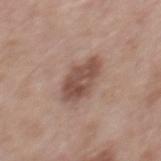<lesion>
<lighting>white-light</lighting>
<lesion_size>
  <long_diameter_mm_approx>5.0</long_diameter_mm_approx>
</lesion_size>
<automated_metrics>
  <cielab_L>50</cielab_L>
  <cielab_a>19</cielab_a>
  <cielab_b>24</cielab_b>
  <vs_skin_contrast_norm>8.5</vs_skin_contrast_norm>
  <border_irregularity_0_10>2.5</border_irregularity_0_10>
  <color_variation_0_10>3.5</color_variation_0_10>
  <peripheral_color_asymmetry>1.5</peripheral_color_asymmetry>
</automated_metrics>
<site>mid back</site>
<patient>
  <sex>male</sex>
  <age_approx>55</age_approx>
</patient>
<image>
  <source>total-body photography crop</source>
  <field_of_view_mm>15</field_of_view_mm>
</image>
</lesion>